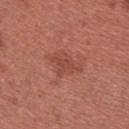No biopsy was performed on this lesion — it was imaged during a full skin examination and was not determined to be concerning.
Captured under white-light illumination.
The lesion is located on the back.
The total-body-photography lesion software estimated an average lesion color of about L≈47 a*≈26 b*≈28 (CIELAB) and a normalized lesion–skin contrast near 5.5. And it measured a border-irregularity index near 5/10, a within-lesion color-variation index near 1.5/10, and peripheral color asymmetry of about 0.5.
A close-up tile cropped from a whole-body skin photograph, about 15 mm across.
The subject is a female aged 23–27.